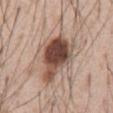notes: no biopsy performed (imaged during a skin exam)
image source: ~15 mm tile from a whole-body skin photo
lesion diameter: ~6.5 mm (longest diameter)
anatomic site: the abdomen
automated metrics: a lesion area of about 20 mm² and a symmetry-axis asymmetry near 0.35; roughly 18 lightness units darker than nearby skin and a normalized lesion–skin contrast near 12; a border-irregularity index near 3.5/10, a within-lesion color-variation index near 9/10, and a peripheral color-asymmetry measure near 2.5
patient: male, in their mid-50s